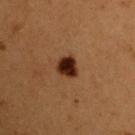Q: Is there a histopathology result?
A: catalogued during a skin exam; not biopsied
Q: How was this image acquired?
A: ~15 mm crop, total-body skin-cancer survey
Q: What is the anatomic site?
A: the right upper arm
Q: Patient demographics?
A: male, aged 48 to 52